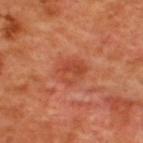biopsy_status: not biopsied; imaged during a skin examination
image:
  source: total-body photography crop
  field_of_view_mm: 15
patient:
  sex: male
  age_approx: 50
site: back